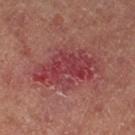Impression:
Imaged during a routine full-body skin examination; the lesion was not biopsied and no histopathology is available.
Acquisition and patient details:
Located on the left thigh. About 7.5 mm across. A 15 mm close-up extracted from a 3D total-body photography capture. A female patient.